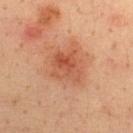Longest diameter approximately 4 mm. A 15 mm crop from a total-body photograph taken for skin-cancer surveillance. A male patient, aged approximately 35. The tile uses cross-polarized illumination. From the upper back.